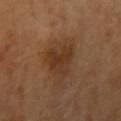Impression:
Recorded during total-body skin imaging; not selected for excision or biopsy.
Clinical summary:
Captured under cross-polarized illumination. The lesion's longest dimension is about 4 mm. A 15 mm crop from a total-body photograph taken for skin-cancer surveillance. The lesion-visualizer software estimated two-axis asymmetry of about 0.4. The software also gave an average lesion color of about L≈35 a*≈20 b*≈31 (CIELAB). And it measured border irregularity of about 4.5 on a 0–10 scale, internal color variation of about 3.5 on a 0–10 scale, and a peripheral color-asymmetry measure near 1.5. A female patient approximately 60 years of age. The lesion is on the left arm.